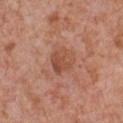notes — imaged on a skin check; not biopsied
patient — male, aged approximately 65
body site — the chest
image — total-body-photography crop, ~15 mm field of view